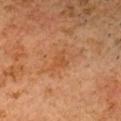Findings:
- notes: no biopsy performed (imaged during a skin exam)
- patient: male, aged 58–62
- anatomic site: the head or neck
- size: about 2.5 mm
- tile lighting: cross-polarized illumination
- image source: total-body-photography crop, ~15 mm field of view